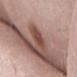Assessment:
The lesion was photographed on a routine skin check and not biopsied; there is no pathology result.